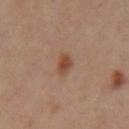{"biopsy_status": "not biopsied; imaged during a skin examination", "image": {"source": "total-body photography crop", "field_of_view_mm": 15}, "patient": {"sex": "female", "age_approx": 45}, "automated_metrics": {"area_mm2_approx": 4.0, "eccentricity": 0.7, "nevus_likeness_0_100": 95, "lesion_detection_confidence_0_100": 100}, "lighting": "cross-polarized", "lesion_size": {"long_diameter_mm_approx": 2.5}, "site": "left upper arm"}